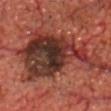Q: Was this lesion biopsied?
A: no biopsy performed (imaged during a skin exam)
Q: What did automated image analysis measure?
A: a footprint of about 46 mm², an outline eccentricity of about 0.85 (0 = round, 1 = elongated), and two-axis asymmetry of about 0.55; border irregularity of about 9 on a 0–10 scale, internal color variation of about 10 on a 0–10 scale, and peripheral color asymmetry of about 3.5
Q: Who is the patient?
A: male, roughly 70 years of age
Q: What lighting was used for the tile?
A: cross-polarized
Q: What kind of image is this?
A: 15 mm crop, total-body photography
Q: What is the lesion's diameter?
A: about 14 mm
Q: Where on the body is the lesion?
A: the chest